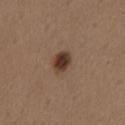Assessment:
Recorded during total-body skin imaging; not selected for excision or biopsy.
Image and clinical context:
From the mid back. The patient is a female aged around 40. This image is a 15 mm lesion crop taken from a total-body photograph.